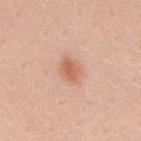Assessment:
Captured during whole-body skin photography for melanoma surveillance; the lesion was not biopsied.